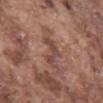Captured during whole-body skin photography for melanoma surveillance; the lesion was not biopsied.
The recorded lesion diameter is about 4 mm.
A male subject in their mid-70s.
Automated image analysis of the tile measured an area of roughly 5 mm² and an outline eccentricity of about 0.85 (0 = round, 1 = elongated). The analysis additionally found a border-irregularity index near 7.5/10 and radial color variation of about 0.5. It also reported a classifier nevus-likeness of about 0/100 and a lesion-detection confidence of about 70/100.
This is a white-light tile.
On the mid back.
A region of skin cropped from a whole-body photographic capture, roughly 15 mm wide.